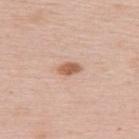  biopsy_status: not biopsied; imaged during a skin examination
  patient:
    sex: female
    age_approx: 65
  image:
    source: total-body photography crop
    field_of_view_mm: 15
  automated_metrics:
    area_mm2_approx: 3.5
    eccentricity: 0.8
    shape_asymmetry: 0.2
    nevus_likeness_0_100: 95
    lesion_detection_confidence_0_100: 100
  lighting: white-light
  site: upper back